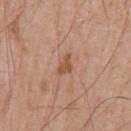Notes:
– follow-up — catalogued during a skin exam; not biopsied